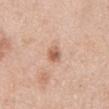| feature | finding |
|---|---|
| follow-up | imaged on a skin check; not biopsied |
| image source | total-body-photography crop, ~15 mm field of view |
| location | the mid back |
| patient | male, aged approximately 60 |
| tile lighting | white-light illumination |
| lesion size | ≈3 mm |